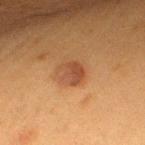• workup: total-body-photography surveillance lesion; no biopsy
• subject: female, approximately 50 years of age
• image: ~15 mm tile from a whole-body skin photo
• illumination: cross-polarized illumination
• automated metrics: a nevus-likeness score of about 50/100
• diameter: about 3 mm
• body site: the upper back